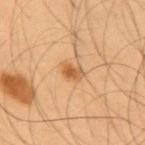{"biopsy_status": "not biopsied; imaged during a skin examination", "patient": {"sex": "male", "age_approx": 55}, "automated_metrics": {"area_mm2_approx": 3.5, "eccentricity": 0.75, "shape_asymmetry": 0.25, "border_irregularity_0_10": 2.5, "color_variation_0_10": 4.0, "peripheral_color_asymmetry": 1.0}, "lighting": "cross-polarized", "site": "mid back", "lesion_size": {"long_diameter_mm_approx": 2.5}, "image": {"source": "total-body photography crop", "field_of_view_mm": 15}}